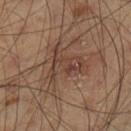Clinical summary: The lesion is located on the left lower leg. A 15 mm close-up tile from a total-body photography series done for melanoma screening. The total-body-photography lesion software estimated two-axis asymmetry of about 0.6. And it measured a mean CIELAB color near L≈42 a*≈17 b*≈25, a lesion–skin lightness drop of about 7, and a normalized border contrast of about 6. The software also gave border irregularity of about 8.5 on a 0–10 scale, internal color variation of about 5 on a 0–10 scale, and a peripheral color-asymmetry measure near 1.5. Captured under cross-polarized illumination. The lesion's longest dimension is about 6 mm. A male patient aged around 40.